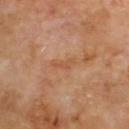biopsy status = catalogued during a skin exam; not biopsied
image-analysis metrics = a symmetry-axis asymmetry near 0.55; a mean CIELAB color near L≈52 a*≈23 b*≈34 and roughly 6 lightness units darker than nearby skin; a border-irregularity rating of about 6/10, internal color variation of about 0 on a 0–10 scale, and radial color variation of about 0
illumination = cross-polarized illumination
patient = male, aged approximately 70
imaging modality = ~15 mm tile from a whole-body skin photo
size = ≈3 mm
anatomic site = the upper back